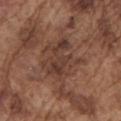The lesion was photographed on a routine skin check and not biopsied; there is no pathology result. A roughly 15 mm field-of-view crop from a total-body skin photograph. The lesion is located on the left upper arm. The subject is a male aged around 75. Automated image analysis of the tile measured a footprint of about 13 mm² and an eccentricity of roughly 0.6. The analysis additionally found a nevus-likeness score of about 0/100 and a lesion-detection confidence of about 95/100. Longest diameter approximately 5 mm.